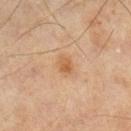Impression: The lesion was tiled from a total-body skin photograph and was not biopsied. Image and clinical context: The recorded lesion diameter is about 2.5 mm. A lesion tile, about 15 mm wide, cut from a 3D total-body photograph. A male patient, aged around 60. On the left lower leg.